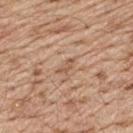This lesion was catalogued during total-body skin photography and was not selected for biopsy.